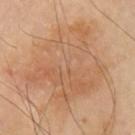* notes — total-body-photography surveillance lesion; no biopsy
* anatomic site — the left upper arm
* lighting — cross-polarized
* subject — male, approximately 65 years of age
* automated lesion analysis — a lesion area of about 55 mm² and an eccentricity of roughly 0.5; a mean CIELAB color near L≈60 a*≈20 b*≈35, a lesion–skin lightness drop of about 7, and a normalized border contrast of about 5; border irregularity of about 5.5 on a 0–10 scale and a within-lesion color-variation index near 5/10; a nevus-likeness score of about 0/100 and lesion-presence confidence of about 100/100
* lesion size — about 10 mm
* acquisition — 15 mm crop, total-body photography